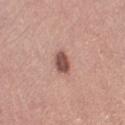workup: imaged on a skin check; not biopsied | lesion size: about 3 mm | image source: ~15 mm tile from a whole-body skin photo | site: the right lower leg | subject: female, aged around 65 | lighting: white-light illumination | TBP lesion metrics: an eccentricity of roughly 0.8; an average lesion color of about L≈51 a*≈22 b*≈24 (CIELAB), a lesion–skin lightness drop of about 15, and a normalized lesion–skin contrast near 10; a border-irregularity index near 2.5/10, a color-variation rating of about 2.5/10, and peripheral color asymmetry of about 1; a lesion-detection confidence of about 100/100.